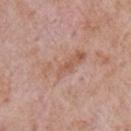Findings:
- notes — total-body-photography surveillance lesion; no biopsy
- TBP lesion metrics — a lesion color around L≈58 a*≈20 b*≈28 in CIELAB, roughly 7 lightness units darker than nearby skin, and a normalized border contrast of about 5; a nevus-likeness score of about 0/100
- acquisition — 15 mm crop, total-body photography
- tile lighting — white-light
- anatomic site — the right upper arm
- diameter — about 5.5 mm
- subject — male, in their mid- to late 50s